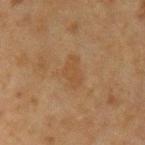Q: Was this lesion biopsied?
A: imaged on a skin check; not biopsied
Q: Lesion location?
A: the left upper arm
Q: What did automated image analysis measure?
A: a lesion–skin lightness drop of about 4 and a lesion-to-skin contrast of about 5 (normalized; higher = more distinct); a border-irregularity rating of about 3/10, internal color variation of about 1 on a 0–10 scale, and a peripheral color-asymmetry measure near 0.5
Q: How was this image acquired?
A: ~15 mm crop, total-body skin-cancer survey
Q: Patient demographics?
A: male, aged 48–52